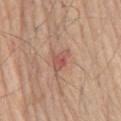The subject is a male aged 78 to 82. The lesion is located on the lower back. Automated tile analysis of the lesion measured an area of roughly 4 mm². The analysis additionally found a border-irregularity rating of about 4/10 and a within-lesion color-variation index near 3.5/10. And it measured a nevus-likeness score of about 0/100 and lesion-presence confidence of about 100/100. A close-up tile cropped from a whole-body skin photograph, about 15 mm across. Captured under white-light illumination. The recorded lesion diameter is about 3 mm.